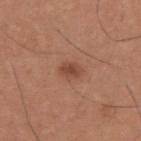This lesion was catalogued during total-body skin photography and was not selected for biopsy.
A region of skin cropped from a whole-body photographic capture, roughly 15 mm wide.
Located on the back.
A male patient, roughly 35 years of age.